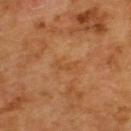The patient is a male aged 63–67.
A region of skin cropped from a whole-body photographic capture, roughly 15 mm wide.
Located on the upper back.